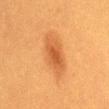Q: Is there a histopathology result?
A: total-body-photography surveillance lesion; no biopsy
Q: How was this image acquired?
A: 15 mm crop, total-body photography
Q: What lighting was used for the tile?
A: cross-polarized
Q: Lesion location?
A: the mid back
Q: Patient demographics?
A: female, aged approximately 40
Q: Lesion size?
A: ~6 mm (longest diameter)
Q: What did automated image analysis measure?
A: an area of roughly 11 mm², an eccentricity of roughly 0.9, and a symmetry-axis asymmetry near 0.2; a border-irregularity rating of about 2.5/10 and a color-variation rating of about 3.5/10; a nevus-likeness score of about 100/100